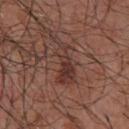{
  "biopsy_status": "not biopsied; imaged during a skin examination",
  "automated_metrics": {
    "cielab_L": 36,
    "cielab_a": 20,
    "cielab_b": 23,
    "vs_skin_contrast_norm": 7.0,
    "border_irregularity_0_10": 6.5,
    "color_variation_0_10": 6.0
  },
  "lighting": "white-light",
  "patient": {
    "sex": "male",
    "age_approx": 55
  },
  "lesion_size": {
    "long_diameter_mm_approx": 6.0
  },
  "site": "chest",
  "image": {
    "source": "total-body photography crop",
    "field_of_view_mm": 15
  }
}